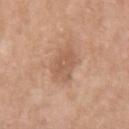The lesion was photographed on a routine skin check and not biopsied; there is no pathology result.